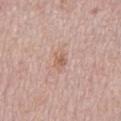Impression: Captured during whole-body skin photography for melanoma surveillance; the lesion was not biopsied. Background: A male patient, aged approximately 75. An algorithmic analysis of the crop reported a footprint of about 4 mm² and a shape eccentricity near 0.8. The lesion is located on the back. A 15 mm crop from a total-body photograph taken for skin-cancer surveillance. The tile uses white-light illumination.